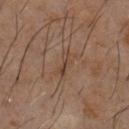- lesion size: about 3 mm
- imaging modality: 15 mm crop, total-body photography
- illumination: cross-polarized illumination
- automated lesion analysis: a mean CIELAB color near L≈38 a*≈15 b*≈25, about 7 CIELAB-L* units darker than the surrounding skin, and a normalized lesion–skin contrast near 6; a border-irregularity index near 6/10 and a peripheral color-asymmetry measure near 0; an automated nevus-likeness rating near 0 out of 100 and a lesion-detection confidence of about 55/100
- subject: male, in their 60s
- body site: the chest